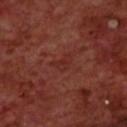Impression:
Recorded during total-body skin imaging; not selected for excision or biopsy.
Acquisition and patient details:
A male patient, aged around 70. Automated image analysis of the tile measured a mean CIELAB color near L≈28 a*≈26 b*≈24 and a lesion–skin lightness drop of about 4. And it measured a border-irregularity index near 5/10, a within-lesion color-variation index near 2/10, and a peripheral color-asymmetry measure near 0.5. The lesion's longest dimension is about 2.5 mm. This is a cross-polarized tile. On the back. This image is a 15 mm lesion crop taken from a total-body photograph.